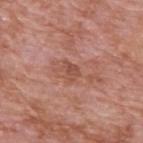Clinical impression: This lesion was catalogued during total-body skin photography and was not selected for biopsy. Context: The lesion is located on the upper back. A close-up tile cropped from a whole-body skin photograph, about 15 mm across. A male patient, about 60 years old.